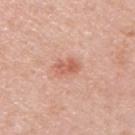{
  "biopsy_status": "not biopsied; imaged during a skin examination",
  "image": {
    "source": "total-body photography crop",
    "field_of_view_mm": 15
  },
  "lighting": "white-light",
  "patient": {
    "sex": "male",
    "age_approx": 45
  },
  "site": "upper back"
}